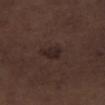No biopsy was performed on this lesion — it was imaged during a full skin examination and was not determined to be concerning. Cropped from a total-body skin-imaging series; the visible field is about 15 mm. A male subject approximately 70 years of age. Longest diameter approximately 2.5 mm. On the right lower leg. Automated image analysis of the tile measured an average lesion color of about L≈23 a*≈13 b*≈16 (CIELAB), a lesion–skin lightness drop of about 6, and a normalized lesion–skin contrast near 8. And it measured a border-irregularity rating of about 2.5/10, internal color variation of about 1.5 on a 0–10 scale, and peripheral color asymmetry of about 0.5. Imaged with white-light lighting.